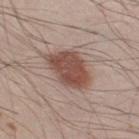workup: imaged on a skin check; not biopsied | body site: the right thigh | patient: male, aged 23 to 27 | image source: ~15 mm tile from a whole-body skin photo.